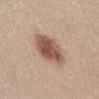subject=female, aged around 40 | size=~5.5 mm (longest diameter) | tile lighting=white-light illumination | anatomic site=the abdomen | image=~15 mm tile from a whole-body skin photo | automated metrics=border irregularity of about 1.5 on a 0–10 scale, a color-variation rating of about 5/10, and radial color variation of about 1.5; a nevus-likeness score of about 100/100.